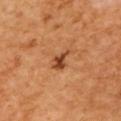The lesion was tiled from a total-body skin photograph and was not biopsied. Automated image analysis of the tile measured an area of roughly 3.5 mm², an eccentricity of roughly 0.75, and two-axis asymmetry of about 0.35. And it measured a border-irregularity index near 3.5/10, a color-variation rating of about 3/10, and peripheral color asymmetry of about 1. A close-up tile cropped from a whole-body skin photograph, about 15 mm across. A female subject. Located on the upper back. The tile uses cross-polarized illumination. Measured at roughly 2.5 mm in maximum diameter.